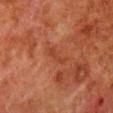Q: Was a biopsy performed?
A: no biopsy performed (imaged during a skin exam)
Q: Lesion location?
A: the right lower leg
Q: What is the imaging modality?
A: 15 mm crop, total-body photography
Q: What lighting was used for the tile?
A: cross-polarized illumination
Q: Lesion size?
A: ~3 mm (longest diameter)
Q: Patient demographics?
A: male, about 80 years old